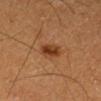Impression: Part of a total-body skin-imaging series; this lesion was reviewed on a skin check and was not flagged for biopsy. Clinical summary: On the left thigh. The lesion's longest dimension is about 3 mm. A male subject about 60 years old. Automated tile analysis of the lesion measured a lesion color around L≈35 a*≈23 b*≈34 in CIELAB, a lesion–skin lightness drop of about 11, and a lesion-to-skin contrast of about 9.5 (normalized; higher = more distinct). It also reported a border-irregularity rating of about 2.5/10 and a within-lesion color-variation index near 3.5/10. The analysis additionally found a nevus-likeness score of about 95/100 and a lesion-detection confidence of about 100/100. Imaged with cross-polarized lighting. A 15 mm crop from a total-body photograph taken for skin-cancer surveillance.